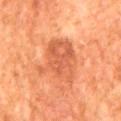Part of a total-body skin-imaging series; this lesion was reviewed on a skin check and was not flagged for biopsy.
An algorithmic analysis of the crop reported a border-irregularity rating of about 4.5/10, internal color variation of about 3.5 on a 0–10 scale, and radial color variation of about 1. And it measured a lesion-detection confidence of about 100/100.
A region of skin cropped from a whole-body photographic capture, roughly 15 mm wide.
From the back.
The tile uses cross-polarized illumination.
The patient is a male aged approximately 80.